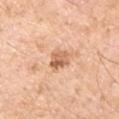This lesion was catalogued during total-body skin photography and was not selected for biopsy. Captured under white-light illumination. A 15 mm close-up extracted from a 3D total-body photography capture. A male patient, aged 68 to 72. From the front of the torso.